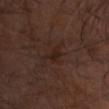  biopsy_status: not biopsied; imaged during a skin examination
  image:
    source: total-body photography crop
    field_of_view_mm: 15
  site: head or neck
  patient:
    sex: male
    age_approx: 60